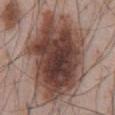Findings:
• location · the front of the torso
• diameter · ≈11 mm
• image source · ~15 mm crop, total-body skin-cancer survey
• subject · male, approximately 55 years of age